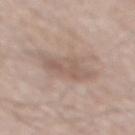biopsy status: total-body-photography surveillance lesion; no biopsy | lesion diameter: about 5.5 mm | image-analysis metrics: an area of roughly 12 mm², an outline eccentricity of about 0.8 (0 = round, 1 = elongated), and a shape-asymmetry score of about 0.3 (0 = symmetric); a lesion color around L≈57 a*≈15 b*≈24 in CIELAB, a lesion–skin lightness drop of about 7, and a normalized border contrast of about 5 | body site: the back | tile lighting: white-light | patient: male, aged 53 to 57 | image source: ~15 mm tile from a whole-body skin photo.